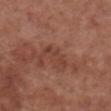notes: catalogued during a skin exam; not biopsied
image: ~15 mm tile from a whole-body skin photo
lesion size: about 4 mm
site: the front of the torso
patient: female, aged 73 to 77